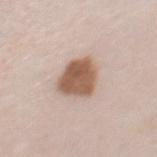<tbp_lesion>
<biopsy_status>not biopsied; imaged during a skin examination</biopsy_status>
<lesion_size>
  <long_diameter_mm_approx>4.5</long_diameter_mm_approx>
</lesion_size>
<automated_metrics>
  <border_irregularity_0_10>2.0</border_irregularity_0_10>
  <color_variation_0_10>3.5</color_variation_0_10>
</automated_metrics>
<lighting>white-light</lighting>
<image>
  <source>total-body photography crop</source>
  <field_of_view_mm>15</field_of_view_mm>
</image>
<site>chest</site>
<patient>
  <sex>female</sex>
  <age_approx>45</age_approx>
</patient>
</tbp_lesion>